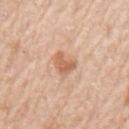<record>
  <automated_metrics>
    <area_mm2_approx>5.5</area_mm2_approx>
    <eccentricity>0.7</eccentricity>
    <shape_asymmetry>0.35</shape_asymmetry>
    <cielab_L>63</cielab_L>
    <cielab_a>21</cielab_a>
    <cielab_b>34</cielab_b>
    <vs_skin_darker_L>10.0</vs_skin_darker_L>
    <vs_skin_contrast_norm>7.0</vs_skin_contrast_norm>
    <nevus_likeness_0_100>30</nevus_likeness_0_100>
    <lesion_detection_confidence_0_100>100</lesion_detection_confidence_0_100>
  </automated_metrics>
  <site>arm</site>
  <lighting>white-light</lighting>
  <image>
    <source>total-body photography crop</source>
    <field_of_view_mm>15</field_of_view_mm>
  </image>
  <patient>
    <sex>male</sex>
    <age_approx>65</age_approx>
  </patient>
</record>